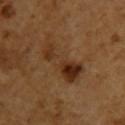Assessment:
Imaged during a routine full-body skin examination; the lesion was not biopsied and no histopathology is available.